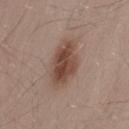This lesion was catalogued during total-body skin photography and was not selected for biopsy.
A lesion tile, about 15 mm wide, cut from a 3D total-body photograph.
An algorithmic analysis of the crop reported a footprint of about 14 mm², an eccentricity of roughly 0.8, and two-axis asymmetry of about 0.15. The software also gave an average lesion color of about L≈46 a*≈19 b*≈25 (CIELAB), roughly 12 lightness units darker than nearby skin, and a normalized border contrast of about 9. It also reported a border-irregularity rating of about 2/10, internal color variation of about 5 on a 0–10 scale, and a peripheral color-asymmetry measure near 1.5. The software also gave an automated nevus-likeness rating near 100 out of 100 and a lesion-detection confidence of about 100/100.
The lesion is on the lower back.
A male subject aged 53–57.